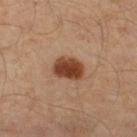notes: total-body-photography surveillance lesion; no biopsy | acquisition: 15 mm crop, total-body photography | lesion size: about 3.5 mm | patient: male, roughly 55 years of age | anatomic site: the right thigh | tile lighting: cross-polarized illumination | automated metrics: a lesion area of about 8 mm², a shape eccentricity near 0.7, and a shape-asymmetry score of about 0.2 (0 = symmetric); border irregularity of about 2 on a 0–10 scale, internal color variation of about 4 on a 0–10 scale, and peripheral color asymmetry of about 1.